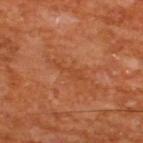Assessment: No biopsy was performed on this lesion — it was imaged during a full skin examination and was not determined to be concerning. Image and clinical context: Located on the back. A male subject, about 65 years old. A lesion tile, about 15 mm wide, cut from a 3D total-body photograph.